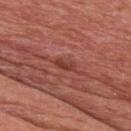Recorded during total-body skin imaging; not selected for excision or biopsy.
Located on the chest.
A 15 mm crop from a total-body photograph taken for skin-cancer surveillance.
The total-body-photography lesion software estimated a within-lesion color-variation index near 0/10. The analysis additionally found an automated nevus-likeness rating near 0 out of 100 and a detector confidence of about 95 out of 100 that the crop contains a lesion.
Longest diameter approximately 2.5 mm.
A male subject roughly 70 years of age.
Imaged with white-light lighting.